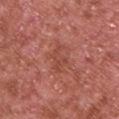Q: Automated lesion metrics?
A: an area of roughly 4.5 mm² and a symmetry-axis asymmetry near 0.4; about 6 CIELAB-L* units darker than the surrounding skin and a lesion-to-skin contrast of about 5 (normalized; higher = more distinct); a classifier nevus-likeness of about 0/100
Q: What is the imaging modality?
A: ~15 mm tile from a whole-body skin photo
Q: What is the lesion's diameter?
A: about 3.5 mm
Q: Patient demographics?
A: male, in their mid- to late 60s
Q: Where on the body is the lesion?
A: the upper back
Q: What lighting was used for the tile?
A: white-light illumination